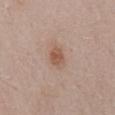- biopsy status: no biopsy performed (imaged during a skin exam)
- diameter: ~2.5 mm (longest diameter)
- imaging modality: 15 mm crop, total-body photography
- automated lesion analysis: an area of roughly 4.5 mm², an eccentricity of roughly 0.5, and two-axis asymmetry of about 0.15; an average lesion color of about L≈54 a*≈20 b*≈28 (CIELAB) and a normalized lesion–skin contrast near 7; a border-irregularity rating of about 1.5/10 and a color-variation rating of about 2.5/10
- illumination: white-light
- site: the mid back
- patient: male, in their mid- to late 50s